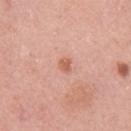The lesion was tiled from a total-body skin photograph and was not biopsied. An algorithmic analysis of the crop reported a nevus-likeness score of about 10/100 and lesion-presence confidence of about 100/100. A female subject aged 38 to 42. A lesion tile, about 15 mm wide, cut from a 3D total-body photograph. The tile uses white-light illumination. The lesion's longest dimension is about 2 mm. On the arm.